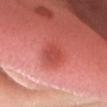The lesion was tiled from a total-body skin photograph and was not biopsied. Captured under white-light illumination. A 15 mm close-up tile from a total-body photography series done for melanoma screening. An algorithmic analysis of the crop reported a lesion area of about 6.5 mm², an eccentricity of roughly 0.6, and two-axis asymmetry of about 0.2. The analysis additionally found a lesion color around L≈51 a*≈38 b*≈32 in CIELAB, a lesion–skin lightness drop of about 9, and a normalized lesion–skin contrast near 6. And it measured a border-irregularity index near 2/10, a within-lesion color-variation index near 2.5/10, and a peripheral color-asymmetry measure near 1. About 3 mm across. The lesion is on the head or neck. The subject is a female aged approximately 45.